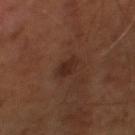Case summary:
– workup · catalogued during a skin exam; not biopsied
– acquisition · ~15 mm crop, total-body skin-cancer survey
– subject · male, in their mid- to late 60s
– illumination · cross-polarized